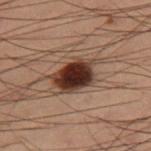Clinical impression:
Recorded during total-body skin imaging; not selected for excision or biopsy.
Context:
The recorded lesion diameter is about 5 mm. From the right thigh. A 15 mm crop from a total-body photograph taken for skin-cancer surveillance. Captured under cross-polarized illumination. A male patient roughly 55 years of age.